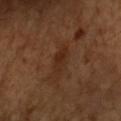Case summary:
- notes — catalogued during a skin exam; not biopsied
- acquisition — 15 mm crop, total-body photography
- location — the front of the torso
- automated lesion analysis — an average lesion color of about L≈27 a*≈20 b*≈28 (CIELAB); border irregularity of about 3 on a 0–10 scale, a within-lesion color-variation index near 2.5/10, and peripheral color asymmetry of about 0.5
- patient — male, about 65 years old
- lesion size — ≈3.5 mm
- tile lighting — cross-polarized